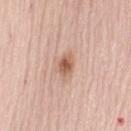{
  "biopsy_status": "not biopsied; imaged during a skin examination"
}